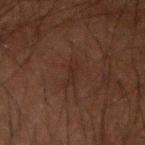  lighting: cross-polarized
  patient:
    sex: male
    age_approx: 50
  automated_metrics:
    area_mm2_approx: 2.5
    eccentricity: 0.9
    border_irregularity_0_10: 3.5
    color_variation_0_10: 0.0
    peripheral_color_asymmetry: 0.0
    nevus_likeness_0_100: 0
    lesion_detection_confidence_0_100: 90
  site: left forearm
  image:
    source: total-body photography crop
    field_of_view_mm: 15